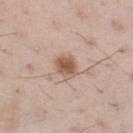This lesion was catalogued during total-body skin photography and was not selected for biopsy.
An algorithmic analysis of the crop reported a shape eccentricity near 0.45 and two-axis asymmetry of about 0.2. It also reported an average lesion color of about L≈56 a*≈19 b*≈29 (CIELAB), a lesion–skin lightness drop of about 12, and a normalized border contrast of about 9. And it measured internal color variation of about 3 on a 0–10 scale. The analysis additionally found a classifier nevus-likeness of about 95/100 and a lesion-detection confidence of about 100/100.
About 3 mm across.
The lesion is located on the left thigh.
A male patient, about 55 years old.
This is a white-light tile.
A 15 mm close-up extracted from a 3D total-body photography capture.